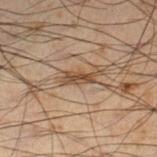Notes:
* workup · no biopsy performed (imaged during a skin exam)
* anatomic site · the leg
* subject · male, aged around 50
* imaging modality · ~15 mm tile from a whole-body skin photo